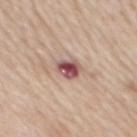Clinical impression:
This lesion was catalogued during total-body skin photography and was not selected for biopsy.
Image and clinical context:
A 15 mm close-up extracted from a 3D total-body photography capture. From the mid back. The recorded lesion diameter is about 3 mm. This is a white-light tile. A male subject about 60 years old.